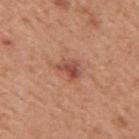Clinical impression: The lesion was photographed on a routine skin check and not biopsied; there is no pathology result. Background: Approximately 3 mm at its widest. A 15 mm crop from a total-body photograph taken for skin-cancer surveillance. An algorithmic analysis of the crop reported an average lesion color of about L≈50 a*≈26 b*≈29 (CIELAB), roughly 11 lightness units darker than nearby skin, and a normalized border contrast of about 7.5. And it measured a lesion-detection confidence of about 100/100. On the right upper arm. A male subject, about 50 years old. This is a white-light tile.